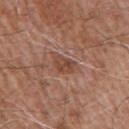lighting: white-light
site: right upper arm
automated_metrics:
  peripheral_color_asymmetry: 1.0
  nevus_likeness_0_100: 10
  lesion_detection_confidence_0_100: 100
lesion_size:
  long_diameter_mm_approx: 3.0
image:
  source: total-body photography crop
  field_of_view_mm: 15
patient:
  sex: male
  age_approx: 75